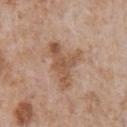No biopsy was performed on this lesion — it was imaged during a full skin examination and was not determined to be concerning. The patient is a male in their mid-60s. The lesion-visualizer software estimated an eccentricity of roughly 0.8. And it measured a mean CIELAB color near L≈54 a*≈19 b*≈31, about 10 CIELAB-L* units darker than the surrounding skin, and a normalized border contrast of about 7. The software also gave a classifier nevus-likeness of about 0/100. From the chest. A 15 mm crop from a total-body photograph taken for skin-cancer surveillance. Captured under white-light illumination. The lesion's longest dimension is about 6 mm.